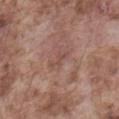Clinical impression: Part of a total-body skin-imaging series; this lesion was reviewed on a skin check and was not flagged for biopsy. Acquisition and patient details: Approximately 3 mm at its widest. The lesion is on the abdomen. Cropped from a total-body skin-imaging series; the visible field is about 15 mm. A male subject roughly 75 years of age. Automated tile analysis of the lesion measured a border-irregularity index near 6.5/10, a color-variation rating of about 0/10, and a peripheral color-asymmetry measure near 0. The software also gave an automated nevus-likeness rating near 0 out of 100. Imaged with white-light lighting.